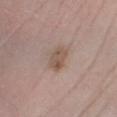Part of a total-body skin-imaging series; this lesion was reviewed on a skin check and was not flagged for biopsy.
The patient is a male aged 58–62.
This is a white-light tile.
About 3.5 mm across.
Located on the right lower leg.
Cropped from a whole-body photographic skin survey; the tile spans about 15 mm.